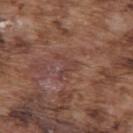Clinical impression:
Recorded during total-body skin imaging; not selected for excision or biopsy.
Background:
A close-up tile cropped from a whole-body skin photograph, about 15 mm across. Measured at roughly 3 mm in maximum diameter. The lesion is on the upper back. Imaged with white-light lighting. The lesion-visualizer software estimated an average lesion color of about L≈41 a*≈20 b*≈23 (CIELAB), about 6 CIELAB-L* units darker than the surrounding skin, and a lesion-to-skin contrast of about 5 (normalized; higher = more distinct). A male subject aged 73–77.